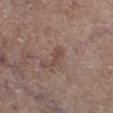  biopsy_status: not biopsied; imaged during a skin examination
  site: left lower leg
  lighting: white-light
  automated_metrics:
    area_mm2_approx: 3.0
    eccentricity: 0.9
    shape_asymmetry: 0.4
  patient:
    sex: male
    age_approx: 65
  lesion_size:
    long_diameter_mm_approx: 2.5
  image:
    source: total-body photography crop
    field_of_view_mm: 15